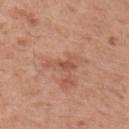Automated tile analysis of the lesion measured a lesion area of about 4 mm² and an outline eccentricity of about 0.9 (0 = round, 1 = elongated). The analysis additionally found an automated nevus-likeness rating near 0 out of 100 and a lesion-detection confidence of about 100/100. Captured under white-light illumination. A close-up tile cropped from a whole-body skin photograph, about 15 mm across. A male patient in their mid-50s. From the right upper arm. About 3.5 mm across.